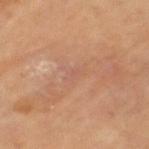<case>
<biopsy_status>not biopsied; imaged during a skin examination</biopsy_status>
<image>
  <source>total-body photography crop</source>
  <field_of_view_mm>15</field_of_view_mm>
</image>
<patient>
  <sex>female</sex>
  <age_approx>70</age_approx>
</patient>
<site>left thigh</site>
</case>